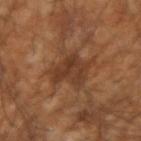Clinical impression: Recorded during total-body skin imaging; not selected for excision or biopsy. Acquisition and patient details: A 15 mm close-up tile from a total-body photography series done for melanoma screening. Approximately 4.5 mm at its widest. The lesion is located on the right forearm. An algorithmic analysis of the crop reported an area of roughly 14 mm², an eccentricity of roughly 0.4, and a shape-asymmetry score of about 0.35 (0 = symmetric). The software also gave a border-irregularity rating of about 5/10 and a peripheral color-asymmetry measure near 1. And it measured an automated nevus-likeness rating near 0 out of 100 and a detector confidence of about 85 out of 100 that the crop contains a lesion. The tile uses cross-polarized illumination. The patient is a male approximately 55 years of age.